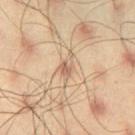{
  "biopsy_status": "not biopsied; imaged during a skin examination",
  "image": {
    "source": "total-body photography crop",
    "field_of_view_mm": 15
  },
  "lesion_size": {
    "long_diameter_mm_approx": 3.0
  },
  "patient": {
    "sex": "male",
    "age_approx": 45
  },
  "site": "left thigh"
}